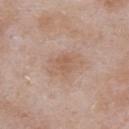{"biopsy_status": "not biopsied; imaged during a skin examination", "lesion_size": {"long_diameter_mm_approx": 4.5}, "image": {"source": "total-body photography crop", "field_of_view_mm": 15}, "automated_metrics": {"eccentricity": 0.8, "shape_asymmetry": 0.25, "border_irregularity_0_10": 3.0}, "lighting": "white-light", "patient": {"sex": "male", "age_approx": 55}, "site": "abdomen"}